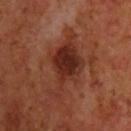biopsy_status: not biopsied; imaged during a skin examination
patient:
  sex: male
  age_approx: 70
image:
  source: total-body photography crop
  field_of_view_mm: 15
automated_metrics:
  border_irregularity_0_10: 6.0
  color_variation_0_10: 5.0
  peripheral_color_asymmetry: 1.5
  lesion_detection_confidence_0_100: 100
lesion_size:
  long_diameter_mm_approx: 8.0
site: chest